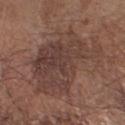{
  "biopsy_status": "not biopsied; imaged during a skin examination",
  "image": {
    "source": "total-body photography crop",
    "field_of_view_mm": 15
  },
  "patient": {
    "sex": "male",
    "age_approx": 70
  },
  "lesion_size": {
    "long_diameter_mm_approx": 10.5
  },
  "site": "right forearm",
  "lighting": "white-light"
}